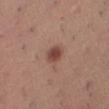follow-up = imaged on a skin check; not biopsied | patient = female, roughly 40 years of age | size = ≈3 mm | anatomic site = the right thigh | image = 15 mm crop, total-body photography.